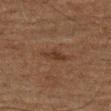Part of a total-body skin-imaging series; this lesion was reviewed on a skin check and was not flagged for biopsy. A male subject, about 75 years old. The lesion's longest dimension is about 3 mm. Imaged with cross-polarized lighting. From the left thigh. Cropped from a total-body skin-imaging series; the visible field is about 15 mm.